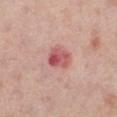Assessment:
The lesion was photographed on a routine skin check and not biopsied; there is no pathology result.
Acquisition and patient details:
Cropped from a total-body skin-imaging series; the visible field is about 15 mm. On the left lower leg. The patient is a female aged approximately 65. Imaged with white-light lighting.